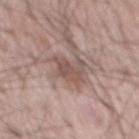The lesion was tiled from a total-body skin photograph and was not biopsied. A male subject in their mid-60s. Longest diameter approximately 4.5 mm. An algorithmic analysis of the crop reported an outline eccentricity of about 0.7 (0 = round, 1 = elongated) and a shape-asymmetry score of about 0.25 (0 = symmetric). The analysis additionally found a mean CIELAB color near L≈53 a*≈17 b*≈22 and roughly 10 lightness units darker than nearby skin. The analysis additionally found a border-irregularity rating of about 4/10 and peripheral color asymmetry of about 1. The analysis additionally found an automated nevus-likeness rating near 0 out of 100 and a detector confidence of about 100 out of 100 that the crop contains a lesion. A roughly 15 mm field-of-view crop from a total-body skin photograph. Captured under white-light illumination. On the mid back.